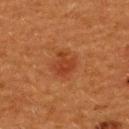workup: no biopsy performed (imaged during a skin exam)
image: ~15 mm crop, total-body skin-cancer survey
image-analysis metrics: a classifier nevus-likeness of about 10/100
site: the upper back
subject: female, aged around 40
illumination: cross-polarized
lesion diameter: about 3 mm